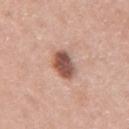{"biopsy_status": "not biopsied; imaged during a skin examination", "image": {"source": "total-body photography crop", "field_of_view_mm": 15}, "lesion_size": {"long_diameter_mm_approx": 3.5}, "site": "arm", "patient": {"sex": "male", "age_approx": 60}}